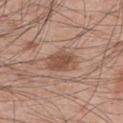The lesion was tiled from a total-body skin photograph and was not biopsied.
Measured at roughly 4 mm in maximum diameter.
The lesion-visualizer software estimated about 10 CIELAB-L* units darker than the surrounding skin and a normalized lesion–skin contrast near 7.5. It also reported a border-irregularity rating of about 2/10 and radial color variation of about 1. And it measured a lesion-detection confidence of about 100/100.
From the left upper arm.
Cropped from a whole-body photographic skin survey; the tile spans about 15 mm.
A male patient in their mid-40s.